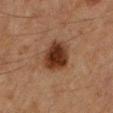<lesion>
<biopsy_status>not biopsied; imaged during a skin examination</biopsy_status>
<patient>
  <sex>male</sex>
  <age_approx>85+</age_approx>
</patient>
<image>
  <source>total-body photography crop</source>
  <field_of_view_mm>15</field_of_view_mm>
</image>
<automated_metrics>
  <area_mm2_approx>12.0</area_mm2_approx>
  <shape_asymmetry>0.15</shape_asymmetry>
  <cielab_L>30</cielab_L>
  <cielab_a>19</cielab_a>
  <cielab_b>27</cielab_b>
  <vs_skin_darker_L>14.0</vs_skin_darker_L>
</automated_metrics>
<site>leg</site>
</lesion>